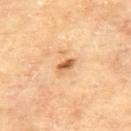follow-up = imaged on a skin check; not biopsied.